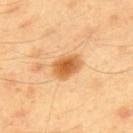Assessment:
No biopsy was performed on this lesion — it was imaged during a full skin examination and was not determined to be concerning.
Background:
A lesion tile, about 15 mm wide, cut from a 3D total-body photograph. A male patient, aged 43–47. On the mid back. The lesion's longest dimension is about 3.5 mm. The total-body-photography lesion software estimated a lesion area of about 8 mm², a shape eccentricity near 0.7, and two-axis asymmetry of about 0.15. The analysis additionally found border irregularity of about 1.5 on a 0–10 scale and radial color variation of about 1.5.